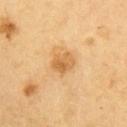The lesion was tiled from a total-body skin photograph and was not biopsied. A 15 mm close-up extracted from a 3D total-body photography capture. Automated image analysis of the tile measured an outline eccentricity of about 0.6 (0 = round, 1 = elongated) and a shape-asymmetry score of about 0.2 (0 = symmetric). The analysis additionally found a nevus-likeness score of about 35/100. The tile uses cross-polarized illumination. The recorded lesion diameter is about 3 mm. The lesion is on the right upper arm. A male patient, aged around 60.